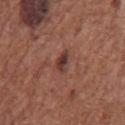The lesion was tiled from a total-body skin photograph and was not biopsied. The lesion is located on the chest. The tile uses white-light illumination. Automated image analysis of the tile measured a footprint of about 3.5 mm², an eccentricity of roughly 0.8, and two-axis asymmetry of about 0.3. A region of skin cropped from a whole-body photographic capture, roughly 15 mm wide. The subject is a male aged around 75. Measured at roughly 2.5 mm in maximum diameter.